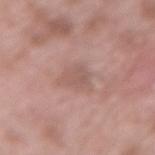Background:
A 15 mm close-up tile from a total-body photography series done for melanoma screening. Located on the lower back. A male subject, approximately 55 years of age. Captured under white-light illumination.